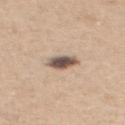On the mid back. A lesion tile, about 15 mm wide, cut from a 3D total-body photograph. The recorded lesion diameter is about 3 mm. Automated tile analysis of the lesion measured a lesion area of about 5.5 mm², an outline eccentricity of about 0.7 (0 = round, 1 = elongated), and a symmetry-axis asymmetry near 0.15. And it measured an average lesion color of about L≈54 a*≈14 b*≈23 (CIELAB) and a lesion–skin lightness drop of about 18. The analysis additionally found border irregularity of about 2 on a 0–10 scale, internal color variation of about 5 on a 0–10 scale, and radial color variation of about 1.5. Captured under white-light illumination. The patient is a female aged 43 to 47.